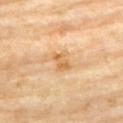Findings:
• biopsy status — imaged on a skin check; not biopsied
• image-analysis metrics — a border-irregularity index near 3/10, a within-lesion color-variation index near 4/10, and a peripheral color-asymmetry measure near 1; a classifier nevus-likeness of about 0/100
• imaging modality — ~15 mm crop, total-body skin-cancer survey
• patient — female, aged 73 to 77
• location — the chest
• diameter — ≈3 mm
• lighting — cross-polarized illumination